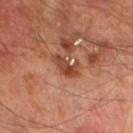Q: Is there a histopathology result?
A: total-body-photography surveillance lesion; no biopsy
Q: What lighting was used for the tile?
A: cross-polarized illumination
Q: Who is the patient?
A: male, aged approximately 70
Q: What kind of image is this?
A: ~15 mm crop, total-body skin-cancer survey
Q: What is the anatomic site?
A: the leg
Q: Lesion size?
A: ~3 mm (longest diameter)
Q: Automated lesion metrics?
A: an eccentricity of roughly 0.85 and a shape-asymmetry score of about 0.35 (0 = symmetric); a lesion color around L≈43 a*≈25 b*≈33 in CIELAB and a lesion-to-skin contrast of about 8.5 (normalized; higher = more distinct); a within-lesion color-variation index near 3/10 and peripheral color asymmetry of about 0.5